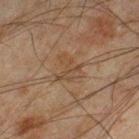Clinical impression: Recorded during total-body skin imaging; not selected for excision or biopsy. Context: A region of skin cropped from a whole-body photographic capture, roughly 15 mm wide. The lesion-visualizer software estimated a lesion area of about 6.5 mm², an eccentricity of roughly 0.6, and two-axis asymmetry of about 0.55. The analysis additionally found a mean CIELAB color near L≈37 a*≈14 b*≈26 and about 5 CIELAB-L* units darker than the surrounding skin. And it measured a border-irregularity index near 7/10, a within-lesion color-variation index near 2.5/10, and a peripheral color-asymmetry measure near 1. On the right lower leg. Measured at roughly 3.5 mm in maximum diameter. A male patient, approximately 45 years of age.